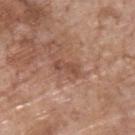Q: Was this lesion biopsied?
A: catalogued during a skin exam; not biopsied
Q: How was this image acquired?
A: total-body-photography crop, ~15 mm field of view
Q: How was the tile lit?
A: white-light
Q: Where on the body is the lesion?
A: the upper back
Q: What are the patient's age and sex?
A: male, approximately 70 years of age